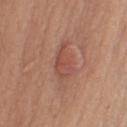Q: Where on the body is the lesion?
A: the arm
Q: What did automated image analysis measure?
A: a shape eccentricity near 0.85; about 7 CIELAB-L* units darker than the surrounding skin; a lesion-detection confidence of about 95/100
Q: Illumination type?
A: white-light
Q: Who is the patient?
A: male, aged 73–77
Q: What is the imaging modality?
A: 15 mm crop, total-body photography